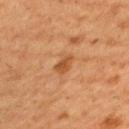The lesion was photographed on a routine skin check and not biopsied; there is no pathology result. Cropped from a total-body skin-imaging series; the visible field is about 15 mm. A female subject aged around 40. The lesion-visualizer software estimated a border-irregularity rating of about 2/10, a color-variation rating of about 1.5/10, and a peripheral color-asymmetry measure near 0.5. The software also gave a nevus-likeness score of about 5/100 and lesion-presence confidence of about 100/100. From the upper back. This is a cross-polarized tile.